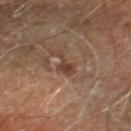The lesion is located on the right lower leg.
A male subject approximately 70 years of age.
A region of skin cropped from a whole-body photographic capture, roughly 15 mm wide.